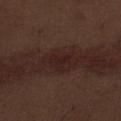{
  "image": {
    "source": "total-body photography crop",
    "field_of_view_mm": 15
  },
  "lighting": "white-light",
  "patient": {
    "sex": "male",
    "age_approx": 70
  },
  "lesion_size": {
    "long_diameter_mm_approx": 2.5
  },
  "site": "abdomen"
}